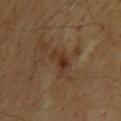* workup: no biopsy performed (imaged during a skin exam)
* location: the upper back
* imaging modality: ~15 mm crop, total-body skin-cancer survey
* patient: male, aged around 65